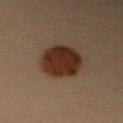lesion_size:
  long_diameter_mm_approx: 5.5
lighting: cross-polarized
image:
  source: total-body photography crop
  field_of_view_mm: 15
site: arm
patient:
  sex: female
  age_approx: 40